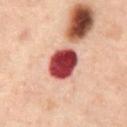* biopsy status — imaged on a skin check; not biopsied
* acquisition — total-body-photography crop, ~15 mm field of view
* anatomic site — the abdomen
* tile lighting — cross-polarized illumination
* image-analysis metrics — an average lesion color of about L≈35 a*≈28 b*≈20 (CIELAB), a lesion–skin lightness drop of about 22, and a normalized lesion–skin contrast near 16.5; a nevus-likeness score of about 0/100 and a lesion-detection confidence of about 100/100
* patient — male, aged approximately 60
* lesion diameter — ~4 mm (longest diameter)